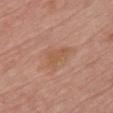{"biopsy_status": "not biopsied; imaged during a skin examination", "site": "chest", "patient": {"sex": "female", "age_approx": 65}, "image": {"source": "total-body photography crop", "field_of_view_mm": 15}}